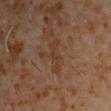Part of a total-body skin-imaging series; this lesion was reviewed on a skin check and was not flagged for biopsy. Automated image analysis of the tile measured a shape eccentricity near 0.95 and two-axis asymmetry of about 0.45. It also reported an average lesion color of about L≈34 a*≈17 b*≈28 (CIELAB), about 5 CIELAB-L* units darker than the surrounding skin, and a normalized border contrast of about 5.5. The analysis additionally found a classifier nevus-likeness of about 0/100 and a lesion-detection confidence of about 95/100. A region of skin cropped from a whole-body photographic capture, roughly 15 mm wide. This is a cross-polarized tile. The lesion is on the arm. The recorded lesion diameter is about 4.5 mm. A male subject, about 60 years old.